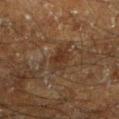<lesion>
<biopsy_status>not biopsied; imaged during a skin examination</biopsy_status>
<site>left lower leg</site>
<image>
  <source>total-body photography crop</source>
  <field_of_view_mm>15</field_of_view_mm>
</image>
<patient>
  <sex>male</sex>
  <age_approx>60</age_approx>
</patient>
<lesion_size>
  <long_diameter_mm_approx>3.5</long_diameter_mm_approx>
</lesion_size>
<lighting>cross-polarized</lighting>
</lesion>